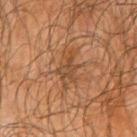patient:
  sex: male
  age_approx: 65
lesion_size:
  long_diameter_mm_approx: 4.5
site: arm
image:
  source: total-body photography crop
  field_of_view_mm: 15